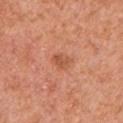Q: Is there a histopathology result?
A: catalogued during a skin exam; not biopsied
Q: Automated lesion metrics?
A: an average lesion color of about L≈55 a*≈28 b*≈36 (CIELAB) and roughly 8 lightness units darker than nearby skin
Q: Who is the patient?
A: male, aged around 55
Q: Lesion location?
A: the front of the torso
Q: Lesion size?
A: ≈2.5 mm
Q: What is the imaging modality?
A: ~15 mm tile from a whole-body skin photo